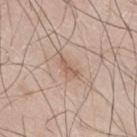The lesion was tiled from a total-body skin photograph and was not biopsied. The total-body-photography lesion software estimated a mean CIELAB color near L≈58 a*≈18 b*≈29, roughly 8 lightness units darker than nearby skin, and a normalized lesion–skin contrast near 6. The software also gave lesion-presence confidence of about 100/100. A 15 mm close-up extracted from a 3D total-body photography capture. The lesion is on the right thigh. A male patient, aged around 60.